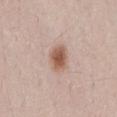No biopsy was performed on this lesion — it was imaged during a full skin examination and was not determined to be concerning. A male subject approximately 25 years of age. Automated image analysis of the tile measured a footprint of about 6.5 mm², an outline eccentricity of about 0.55 (0 = round, 1 = elongated), and a shape-asymmetry score of about 0.3 (0 = symmetric). And it measured a nevus-likeness score of about 100/100. The lesion is on the lower back. A 15 mm close-up extracted from a 3D total-body photography capture. Approximately 3 mm at its widest. The tile uses white-light illumination.